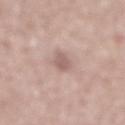Q: Is there a histopathology result?
A: no biopsy performed (imaged during a skin exam)
Q: Automated lesion metrics?
A: an area of roughly 3.5 mm²; a mean CIELAB color near L≈59 a*≈18 b*≈22 and a lesion–skin lightness drop of about 10; border irregularity of about 2.5 on a 0–10 scale, a within-lesion color-variation index near 1/10, and radial color variation of about 0.5
Q: What is the anatomic site?
A: the mid back
Q: How large is the lesion?
A: ~3 mm (longest diameter)
Q: How was the tile lit?
A: white-light illumination
Q: How was this image acquired?
A: ~15 mm tile from a whole-body skin photo
Q: Who is the patient?
A: male, roughly 70 years of age